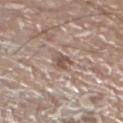biopsy status = imaged on a skin check; not biopsied